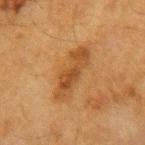follow-up: total-body-photography surveillance lesion; no biopsy | TBP lesion metrics: an average lesion color of about L≈41 a*≈20 b*≈35 (CIELAB) and roughly 8 lightness units darker than nearby skin; a lesion-detection confidence of about 100/100 | anatomic site: the right forearm | diameter: about 6.5 mm | subject: female, aged 53–57 | image: 15 mm crop, total-body photography.